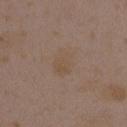Findings:
– site — the arm
– lesion size — ~3.5 mm (longest diameter)
– patient — female, aged 33 to 37
– imaging modality — total-body-photography crop, ~15 mm field of view
– illumination — white-light